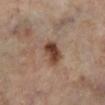Part of a total-body skin-imaging series; this lesion was reviewed on a skin check and was not flagged for biopsy. Longest diameter approximately 3.5 mm. The lesion is located on the left lower leg. The total-body-photography lesion software estimated a lesion area of about 6.5 mm², an outline eccentricity of about 0.7 (0 = round, 1 = elongated), and two-axis asymmetry of about 0.3. A close-up tile cropped from a whole-body skin photograph, about 15 mm across. Captured under cross-polarized illumination. A female patient aged 58 to 62.